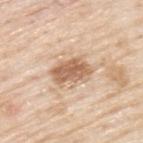Captured during whole-body skin photography for melanoma surveillance; the lesion was not biopsied.
A 15 mm close-up tile from a total-body photography series done for melanoma screening.
About 4.5 mm across.
Captured under white-light illumination.
On the upper back.
A male subject, aged around 80.